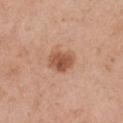- follow-up · total-body-photography surveillance lesion; no biopsy
- site · the right upper arm
- image-analysis metrics · a normalized lesion–skin contrast near 8.5
- illumination · white-light
- patient · male, approximately 75 years of age
- lesion diameter · ≈3.5 mm
- imaging modality · ~15 mm crop, total-body skin-cancer survey